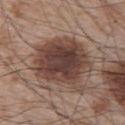No biopsy was performed on this lesion — it was imaged during a full skin examination and was not determined to be concerning. A male subject about 65 years old. The lesion is located on the upper back. A 15 mm close-up extracted from a 3D total-body photography capture.